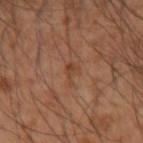follow-up: no biopsy performed (imaged during a skin exam) | imaging modality: ~15 mm tile from a whole-body skin photo | lesion size: about 3 mm | tile lighting: cross-polarized illumination | patient: male, in their mid- to late 50s | body site: the right forearm | image-analysis metrics: an area of roughly 2.5 mm², an outline eccentricity of about 0.9 (0 = round, 1 = elongated), and two-axis asymmetry of about 0.5; an average lesion color of about L≈43 a*≈22 b*≈31 (CIELAB) and about 7 CIELAB-L* units darker than the surrounding skin; a border-irregularity rating of about 6/10, a within-lesion color-variation index near 1/10, and a peripheral color-asymmetry measure near 0.